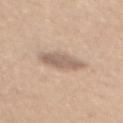No biopsy was performed on this lesion — it was imaged during a full skin examination and was not determined to be concerning. From the upper back. Automated image analysis of the tile measured a lesion color around L≈61 a*≈16 b*≈26 in CIELAB, about 11 CIELAB-L* units darker than the surrounding skin, and a lesion-to-skin contrast of about 7 (normalized; higher = more distinct). Cropped from a whole-body photographic skin survey; the tile spans about 15 mm. The patient is a female roughly 30 years of age.